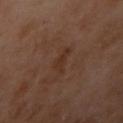Captured during whole-body skin photography for melanoma surveillance; the lesion was not biopsied.
A 15 mm crop from a total-body photograph taken for skin-cancer surveillance.
The patient is a female in their mid-50s.
Captured under cross-polarized illumination.
From the arm.
Measured at roughly 3 mm in maximum diameter.